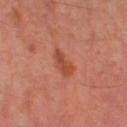Imaged during a routine full-body skin examination; the lesion was not biopsied and no histopathology is available. The lesion is located on the right thigh. The patient is female. A region of skin cropped from a whole-body photographic capture, roughly 15 mm wide. The tile uses cross-polarized illumination.